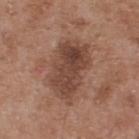{
  "image": {
    "source": "total-body photography crop",
    "field_of_view_mm": 15
  },
  "site": "upper back",
  "patient": {
    "sex": "male",
    "age_approx": 55
  }
}